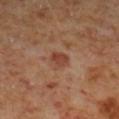The lesion was photographed on a routine skin check and not biopsied; there is no pathology result. A close-up tile cropped from a whole-body skin photograph, about 15 mm across. The subject is a male about 60 years old. From the right lower leg. Captured under cross-polarized illumination.